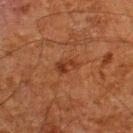  biopsy_status: not biopsied; imaged during a skin examination
  lesion_size:
    long_diameter_mm_approx: 3.0
  site: upper back
  patient:
    sex: male
    age_approx: 60
  automated_metrics:
    cielab_L: 29
    cielab_a: 21
    cielab_b: 28
    vs_skin_darker_L: 6.0
    border_irregularity_0_10: 3.0
    peripheral_color_asymmetry: 1.5
  image:
    source: total-body photography crop
    field_of_view_mm: 15
  lighting: cross-polarized